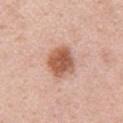<case>
  <biopsy_status>not biopsied; imaged during a skin examination</biopsy_status>
  <patient>
    <sex>male</sex>
    <age_approx>50</age_approx>
  </patient>
  <site>chest</site>
  <automated_metrics>
    <cielab_L>57</cielab_L>
    <cielab_a>24</cielab_a>
    <cielab_b>31</cielab_b>
    <vs_skin_darker_L>15.0</vs_skin_darker_L>
    <vs_skin_contrast_norm>10.0</vs_skin_contrast_norm>
    <color_variation_0_10>4.0</color_variation_0_10>
    <peripheral_color_asymmetry>1.0</peripheral_color_asymmetry>
    <lesion_detection_confidence_0_100>100</lesion_detection_confidence_0_100>
  </automated_metrics>
  <lighting>white-light</lighting>
  <image>
    <source>total-body photography crop</source>
    <field_of_view_mm>15</field_of_view_mm>
  </image>
</case>